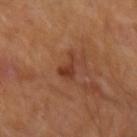  biopsy_status: not biopsied; imaged during a skin examination
  patient:
    sex: male
    age_approx: 65
  lesion_size:
    long_diameter_mm_approx: 3.0
  image:
    source: total-body photography crop
    field_of_view_mm: 15
  lighting: cross-polarized
  site: right upper arm